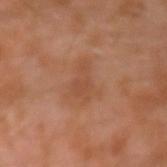follow-up: no biopsy performed (imaged during a skin exam)
image source: ~15 mm crop, total-body skin-cancer survey
automated metrics: a lesion area of about 7.5 mm², an eccentricity of roughly 0.8, and a shape-asymmetry score of about 0.5 (0 = symmetric); about 6 CIELAB-L* units darker than the surrounding skin and a normalized lesion–skin contrast near 5; an automated nevus-likeness rating near 0 out of 100 and a lesion-detection confidence of about 100/100
patient: male, in their 30s
tile lighting: cross-polarized illumination
site: the arm